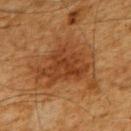{"biopsy_status": "not biopsied; imaged during a skin examination", "patient": {"sex": "male", "age_approx": 60}, "site": "upper back", "image": {"source": "total-body photography crop", "field_of_view_mm": 15}, "lesion_size": {"long_diameter_mm_approx": 8.0}}